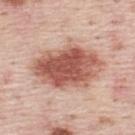Located on the upper back. The total-body-photography lesion software estimated a footprint of about 30 mm² and two-axis asymmetry of about 0.1. And it measured border irregularity of about 2 on a 0–10 scale, a color-variation rating of about 6.5/10, and radial color variation of about 2. It also reported a classifier nevus-likeness of about 95/100. The tile uses white-light illumination. The recorded lesion diameter is about 8.5 mm. A male subject aged approximately 45. A 15 mm close-up tile from a total-body photography series done for melanoma screening.